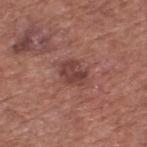<tbp_lesion>
  <biopsy_status>not biopsied; imaged during a skin examination</biopsy_status>
  <lesion_size>
    <long_diameter_mm_approx>3.0</long_diameter_mm_approx>
  </lesion_size>
  <site>upper back</site>
  <patient>
    <sex>male</sex>
    <age_approx>75</age_approx>
  </patient>
  <automated_metrics>
    <border_irregularity_0_10>2.5</border_irregularity_0_10>
    <color_variation_0_10>3.0</color_variation_0_10>
    <peripheral_color_asymmetry>1.5</peripheral_color_asymmetry>
  </automated_metrics>
  <image>
    <source>total-body photography crop</source>
    <field_of_view_mm>15</field_of_view_mm>
  </image>
</tbp_lesion>